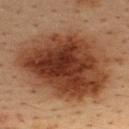Recorded during total-body skin imaging; not selected for excision or biopsy. Automated tile analysis of the lesion measured a footprint of about 70 mm², an outline eccentricity of about 0.65 (0 = round, 1 = elongated), and two-axis asymmetry of about 0.15. The analysis additionally found border irregularity of about 2.5 on a 0–10 scale, internal color variation of about 8 on a 0–10 scale, and radial color variation of about 2.5. It also reported a lesion-detection confidence of about 100/100. The tile uses cross-polarized illumination. The lesion is on the upper back. The patient is a male roughly 35 years of age. A 15 mm crop from a total-body photograph taken for skin-cancer surveillance. Approximately 11 mm at its widest.